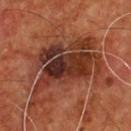{"biopsy_status": "not biopsied; imaged during a skin examination", "site": "front of the torso", "patient": {"sex": "male", "age_approx": 55}, "image": {"source": "total-body photography crop", "field_of_view_mm": 15}}